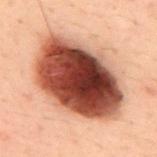Assessment: This lesion was catalogued during total-body skin photography and was not selected for biopsy. Background: From the upper back. The tile uses cross-polarized illumination. Measured at roughly 10 mm in maximum diameter. The subject is a male approximately 40 years of age. Cropped from a whole-body photographic skin survey; the tile spans about 15 mm. Automated tile analysis of the lesion measured a border-irregularity rating of about 1.5/10, a within-lesion color-variation index near 10/10, and peripheral color asymmetry of about 3.5.